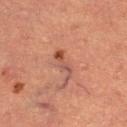{
  "lighting": "cross-polarized",
  "site": "right thigh",
  "patient": {
    "sex": "female",
    "age_approx": 70
  },
  "image": {
    "source": "total-body photography crop",
    "field_of_view_mm": 15
  }
}